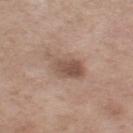Q: Was this lesion biopsied?
A: no biopsy performed (imaged during a skin exam)
Q: How was this image acquired?
A: total-body-photography crop, ~15 mm field of view
Q: Who is the patient?
A: female, in their mid- to late 50s
Q: Automated lesion metrics?
A: a classifier nevus-likeness of about 35/100
Q: Illumination type?
A: white-light illumination
Q: Lesion location?
A: the right thigh